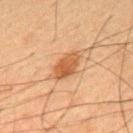Findings:
* notes · total-body-photography surveillance lesion; no biopsy
* imaging modality · 15 mm crop, total-body photography
* image-analysis metrics · a classifier nevus-likeness of about 95/100 and a detector confidence of about 100 out of 100 that the crop contains a lesion
* patient · male, aged approximately 65
* tile lighting · cross-polarized illumination
* size · about 3.5 mm
* location · the mid back